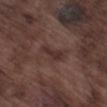{"biopsy_status": "not biopsied; imaged during a skin examination", "image": {"source": "total-body photography crop", "field_of_view_mm": 15}, "lighting": "white-light", "automated_metrics": {"area_mm2_approx": 4.0, "shape_asymmetry": 0.45, "vs_skin_darker_L": 7.0, "vs_skin_contrast_norm": 7.5}, "lesion_size": {"long_diameter_mm_approx": 3.5}, "site": "left thigh", "patient": {"sex": "male", "age_approx": 75}}